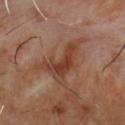The lesion was photographed on a routine skin check and not biopsied; there is no pathology result. Longest diameter approximately 5.5 mm. Located on the chest. A male patient, aged 53 to 57. The lesion-visualizer software estimated a mean CIELAB color near L≈38 a*≈23 b*≈29, roughly 9 lightness units darker than nearby skin, and a normalized border contrast of about 8.5. This image is a 15 mm lesion crop taken from a total-body photograph. Captured under cross-polarized illumination.